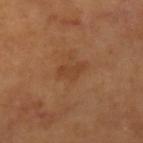notes: no biopsy performed (imaged during a skin exam) | illumination: cross-polarized | patient: female, approximately 55 years of age | image source: 15 mm crop, total-body photography | lesion size: ≈3.5 mm | anatomic site: the right lower leg.